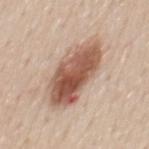Notes:
* tile lighting: white-light illumination
* patient: male, aged approximately 60
* image source: ~15 mm tile from a whole-body skin photo
* body site: the back